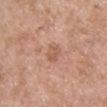Notes:
– notes — total-body-photography surveillance lesion; no biopsy
– site — the chest
– size — ≈2.5 mm
– image — ~15 mm crop, total-body skin-cancer survey
– subject — female, roughly 30 years of age